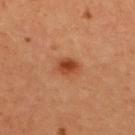lighting: cross-polarized
site: upper back
image:
  source: total-body photography crop
  field_of_view_mm: 15
patient:
  sex: female
  age_approx: 35
lesion_size:
  long_diameter_mm_approx: 3.0